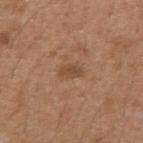| key | value |
|---|---|
| follow-up | total-body-photography surveillance lesion; no biopsy |
| lighting | white-light illumination |
| lesion diameter | about 2.5 mm |
| body site | the left forearm |
| image | ~15 mm tile from a whole-body skin photo |
| subject | female, about 30 years old |